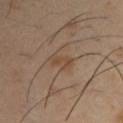The lesion was photographed on a routine skin check and not biopsied; there is no pathology result. On the right upper arm. The lesion-visualizer software estimated a lesion area of about 4.5 mm² and a symmetry-axis asymmetry near 0.2. And it measured roughly 6 lightness units darker than nearby skin. And it measured border irregularity of about 2.5 on a 0–10 scale and internal color variation of about 1.5 on a 0–10 scale. The software also gave a detector confidence of about 100 out of 100 that the crop contains a lesion. Imaged with cross-polarized lighting. The subject is a male in their 40s. A 15 mm close-up tile from a total-body photography series done for melanoma screening. About 3 mm across.